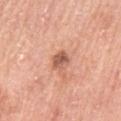Q: Was this lesion biopsied?
A: total-body-photography surveillance lesion; no biopsy
Q: What did automated image analysis measure?
A: a lesion area of about 4.5 mm², an outline eccentricity of about 0.55 (0 = round, 1 = elongated), and two-axis asymmetry of about 0.2; an average lesion color of about L≈58 a*≈25 b*≈30 (CIELAB), a lesion–skin lightness drop of about 13, and a normalized border contrast of about 8; border irregularity of about 2 on a 0–10 scale, a color-variation rating of about 4/10, and radial color variation of about 1.5; a classifier nevus-likeness of about 25/100
Q: What kind of image is this?
A: 15 mm crop, total-body photography
Q: Patient demographics?
A: male, aged 58–62
Q: Where on the body is the lesion?
A: the left upper arm
Q: Illumination type?
A: white-light
Q: Lesion size?
A: ~2.5 mm (longest diameter)